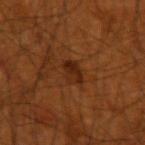{
  "biopsy_status": "not biopsied; imaged during a skin examination",
  "patient": {
    "sex": "male",
    "age_approx": 70
  },
  "image": {
    "source": "total-body photography crop",
    "field_of_view_mm": 15
  },
  "site": "left forearm"
}